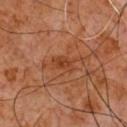{"biopsy_status": "not biopsied; imaged during a skin examination", "patient": {"sex": "male", "age_approx": 60}, "automated_metrics": {"cielab_L": 41, "cielab_a": 26, "cielab_b": 35, "vs_skin_darker_L": 7.0, "vs_skin_contrast_norm": 6.0, "border_irregularity_0_10": 2.5, "color_variation_0_10": 2.5, "peripheral_color_asymmetry": 1.0}, "site": "chest", "image": {"source": "total-body photography crop", "field_of_view_mm": 15}, "lighting": "cross-polarized", "lesion_size": {"long_diameter_mm_approx": 3.0}}